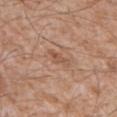Clinical impression: This lesion was catalogued during total-body skin photography and was not selected for biopsy. Clinical summary: A male patient approximately 60 years of age. Automated tile analysis of the lesion measured a peripheral color-asymmetry measure near 0. It also reported an automated nevus-likeness rating near 0 out of 100. The recorded lesion diameter is about 3 mm. Cropped from a total-body skin-imaging series; the visible field is about 15 mm. The tile uses white-light illumination. Located on the mid back.